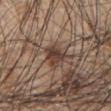<record>
  <biopsy_status>not biopsied; imaged during a skin examination</biopsy_status>
  <site>chest</site>
  <patient>
    <sex>male</sex>
    <age_approx>50</age_approx>
  </patient>
  <lighting>white-light</lighting>
  <lesion_size>
    <long_diameter_mm_approx>3.5</long_diameter_mm_approx>
  </lesion_size>
  <image>
    <source>total-body photography crop</source>
    <field_of_view_mm>15</field_of_view_mm>
  </image>
</record>